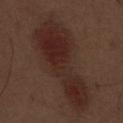Recorded during total-body skin imaging; not selected for excision or biopsy.
A male patient in their 70s.
The tile uses white-light illumination.
The total-body-photography lesion software estimated a footprint of about 35 mm², a shape eccentricity near 0.95, and a symmetry-axis asymmetry near 0.4. It also reported a detector confidence of about 100 out of 100 that the crop contains a lesion.
From the abdomen.
This image is a 15 mm lesion crop taken from a total-body photograph.